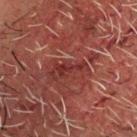The subject is a male aged approximately 65. The tile uses cross-polarized illumination. A lesion tile, about 15 mm wide, cut from a 3D total-body photograph. The lesion is located on the head or neck. Approximately 3.5 mm at its widest.